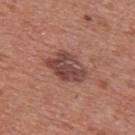Captured during whole-body skin photography for melanoma surveillance; the lesion was not biopsied. The lesion is located on the front of the torso. Cropped from a total-body skin-imaging series; the visible field is about 15 mm. The subject is a male about 45 years old. Longest diameter approximately 5 mm. Automated image analysis of the tile measured an eccentricity of roughly 0.75 and a shape-asymmetry score of about 0.25 (0 = symmetric). The software also gave border irregularity of about 3 on a 0–10 scale and peripheral color asymmetry of about 1.5.